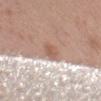Notes:
• workup: imaged on a skin check; not biopsied
• patient: male, aged 48 to 52
• site: the arm
• automated metrics: a lesion area of about 4 mm², an outline eccentricity of about 0.75 (0 = round, 1 = elongated), and a shape-asymmetry score of about 0.2 (0 = symmetric); border irregularity of about 2 on a 0–10 scale, a within-lesion color-variation index near 2.5/10, and peripheral color asymmetry of about 1; an automated nevus-likeness rating near 0 out of 100 and a lesion-detection confidence of about 100/100
• size: ~3 mm (longest diameter)
• image: ~15 mm crop, total-body skin-cancer survey
• illumination: white-light illumination